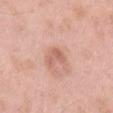Clinical impression:
Imaged during a routine full-body skin examination; the lesion was not biopsied and no histopathology is available.
Clinical summary:
Automated image analysis of the tile measured a shape-asymmetry score of about 0.55 (0 = symmetric). The software also gave a mean CIELAB color near L≈62 a*≈24 b*≈28 and a lesion–skin lightness drop of about 9. The software also gave a classifier nevus-likeness of about 55/100. A roughly 15 mm field-of-view crop from a total-body skin photograph. From the lower back. A male subject approximately 55 years of age. The tile uses white-light illumination.